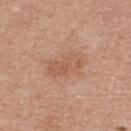No biopsy was performed on this lesion — it was imaged during a full skin examination and was not determined to be concerning.
The lesion's longest dimension is about 5 mm.
An algorithmic analysis of the crop reported an area of roughly 11 mm². And it measured a nevus-likeness score of about 10/100 and a lesion-detection confidence of about 100/100.
Cropped from a whole-body photographic skin survey; the tile spans about 15 mm.
The lesion is on the upper back.
A female patient, about 65 years old.